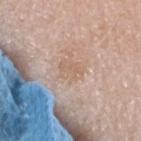Findings:
• biopsy status — no biopsy performed (imaged during a skin exam)
• imaging modality — ~15 mm crop, total-body skin-cancer survey
• lighting — white-light illumination
• size — ~3 mm (longest diameter)
• body site — the head or neck
• subject — female, aged 43–47